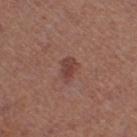A 15 mm crop from a total-body photograph taken for skin-cancer surveillance. From the right thigh. This is a white-light tile. Longest diameter approximately 3 mm. The patient is a female aged approximately 50. Automated tile analysis of the lesion measured a border-irregularity index near 3/10 and a within-lesion color-variation index near 1.5/10. It also reported a nevus-likeness score of about 75/100 and a detector confidence of about 100 out of 100 that the crop contains a lesion.